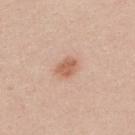Impression:
No biopsy was performed on this lesion — it was imaged during a full skin examination and was not determined to be concerning.
Acquisition and patient details:
The lesion is located on the upper back. A 15 mm close-up extracted from a 3D total-body photography capture. This is a white-light tile. The subject is a female approximately 20 years of age.